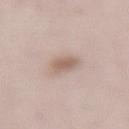biopsy status = catalogued during a skin exam; not biopsied
location = the back
image-analysis metrics = an average lesion color of about L≈60 a*≈16 b*≈25 (CIELAB), about 10 CIELAB-L* units darker than the surrounding skin, and a normalized border contrast of about 6.5; an automated nevus-likeness rating near 90 out of 100 and a lesion-detection confidence of about 100/100
subject = female, aged 28–32
lesion size = ~2.5 mm (longest diameter)
tile lighting = white-light illumination
acquisition = ~15 mm tile from a whole-body skin photo